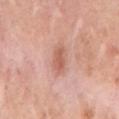biopsy status = imaged on a skin check; not biopsied | image = total-body-photography crop, ~15 mm field of view | anatomic site = the right upper arm | subject = female, aged 58–62 | diameter = about 3 mm | illumination = white-light.